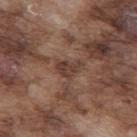  biopsy_status: not biopsied; imaged during a skin examination
  image:
    source: total-body photography crop
    field_of_view_mm: 15
  lighting: white-light
  lesion_size:
    long_diameter_mm_approx: 3.0
  site: back
  patient:
    sex: male
    age_approx: 75